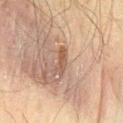Acquisition and patient details: This image is a 15 mm lesion crop taken from a total-body photograph. The lesion is located on the lower back. Imaged with cross-polarized lighting. Longest diameter approximately 3 mm. A male patient, aged around 60.